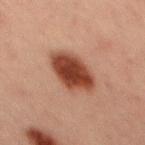follow-up: no biopsy performed (imaged during a skin exam); anatomic site: the mid back; subject: male, approximately 30 years of age; imaging modality: ~15 mm crop, total-body skin-cancer survey.